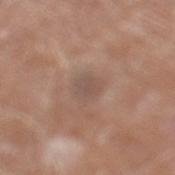<lesion>
  <biopsy_status>not biopsied; imaged during a skin examination</biopsy_status>
  <patient>
    <sex>male</sex>
    <age_approx>80</age_approx>
  </patient>
  <image>
    <source>total-body photography crop</source>
    <field_of_view_mm>15</field_of_view_mm>
  </image>
  <site>left lower leg</site>
</lesion>